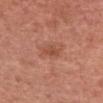<lesion>
<biopsy_status>not biopsied; imaged during a skin examination</biopsy_status>
<image>
  <source>total-body photography crop</source>
  <field_of_view_mm>15</field_of_view_mm>
</image>
<lesion_size>
  <long_diameter_mm_approx>2.5</long_diameter_mm_approx>
</lesion_size>
<patient>
  <sex>female</sex>
  <age_approx>50</age_approx>
</patient>
<lighting>white-light</lighting>
<site>arm</site>
<automated_metrics>
  <nevus_likeness_0_100>10</nevus_likeness_0_100>
</automated_metrics>
</lesion>